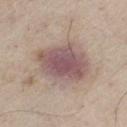  biopsy_status: not biopsied; imaged during a skin examination
  image:
    source: total-body photography crop
    field_of_view_mm: 15
  lesion_size:
    long_diameter_mm_approx: 6.5
  patient:
    sex: male
    age_approx: 60
  site: left thigh